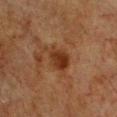The lesion was photographed on a routine skin check and not biopsied; there is no pathology result. Cropped from a whole-body photographic skin survey; the tile spans about 15 mm. A female patient aged approximately 55. The tile uses cross-polarized illumination. The lesion is located on the chest. Measured at roughly 4.5 mm in maximum diameter.